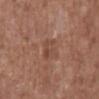Imaged during a routine full-body skin examination; the lesion was not biopsied and no histopathology is available.
Automated tile analysis of the lesion measured a footprint of about 6 mm², an eccentricity of roughly 0.6, and a shape-asymmetry score of about 0.3 (0 = symmetric).
A male subject, aged around 75.
Measured at roughly 3 mm in maximum diameter.
Cropped from a total-body skin-imaging series; the visible field is about 15 mm.
Located on the abdomen.